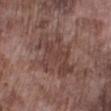Part of a total-body skin-imaging series; this lesion was reviewed on a skin check and was not flagged for biopsy.
A close-up tile cropped from a whole-body skin photograph, about 15 mm across.
The total-body-photography lesion software estimated an area of roughly 19 mm², an outline eccentricity of about 0.7 (0 = round, 1 = elongated), and a symmetry-axis asymmetry near 0.35. The analysis additionally found a mean CIELAB color near L≈41 a*≈18 b*≈22, about 7 CIELAB-L* units darker than the surrounding skin, and a normalized border contrast of about 6. The analysis additionally found a border-irregularity rating of about 6.5/10 and radial color variation of about 1. And it measured a nevus-likeness score of about 0/100 and lesion-presence confidence of about 90/100.
The subject is a male aged 73–77.
From the leg.
This is a white-light tile.